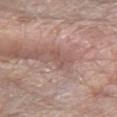biopsy_status: not biopsied; imaged during a skin examination
patient:
  sex: female
  age_approx: 65
site: left forearm
image:
  source: total-body photography crop
  field_of_view_mm: 15